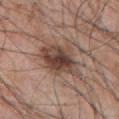This lesion was catalogued during total-body skin photography and was not selected for biopsy.
Located on the chest.
A 15 mm close-up tile from a total-body photography series done for melanoma screening.
Measured at roughly 5 mm in maximum diameter.
A male subject, aged around 70.